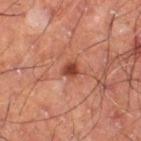biopsy status: no biopsy performed (imaged during a skin exam) | patient: male, approximately 60 years of age | lighting: cross-polarized illumination | image source: ~15 mm tile from a whole-body skin photo | site: the leg | diameter: about 2 mm.